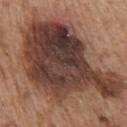Captured under white-light illumination. The lesion's longest dimension is about 13.5 mm. An algorithmic analysis of the crop reported about 17 CIELAB-L* units darker than the surrounding skin and a normalized lesion–skin contrast near 13.5. The software also gave a nevus-likeness score of about 0/100 and lesion-presence confidence of about 80/100. Located on the chest. A 15 mm close-up extracted from a 3D total-body photography capture. The subject is a male aged approximately 75.